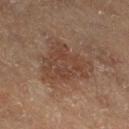The lesion was tiled from a total-body skin photograph and was not biopsied.
The lesion is located on the right thigh.
A 15 mm close-up tile from a total-body photography series done for melanoma screening.
Longest diameter approximately 6 mm.
The subject is a male aged approximately 65.
The tile uses cross-polarized illumination.